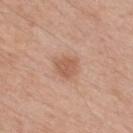Q: Was a biopsy performed?
A: imaged on a skin check; not biopsied
Q: Patient demographics?
A: male, aged approximately 50
Q: Lesion size?
A: ~3 mm (longest diameter)
Q: Lesion location?
A: the mid back
Q: What is the imaging modality?
A: ~15 mm tile from a whole-body skin photo
Q: Illumination type?
A: white-light